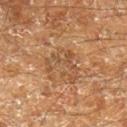biopsy_status: not biopsied; imaged during a skin examination
image:
  source: total-body photography crop
  field_of_view_mm: 15
patient:
  sex: male
  age_approx: 60
lighting: cross-polarized
site: right lower leg
lesion_size:
  long_diameter_mm_approx: 4.5